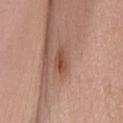Impression:
This lesion was catalogued during total-body skin photography and was not selected for biopsy.
Clinical summary:
A female subject, aged 43 to 47. Cropped from a whole-body photographic skin survey; the tile spans about 15 mm. Automated tile analysis of the lesion measured a footprint of about 4 mm² and an outline eccentricity of about 0.85 (0 = round, 1 = elongated). The software also gave a lesion color around L≈49 a*≈23 b*≈29 in CIELAB, roughly 9 lightness units darker than nearby skin, and a lesion-to-skin contrast of about 7 (normalized; higher = more distinct). Located on the chest.